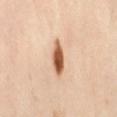This lesion was catalogued during total-body skin photography and was not selected for biopsy.
The lesion is located on the right thigh.
The tile uses cross-polarized illumination.
A female patient, aged 38 to 42.
Automated image analysis of the tile measured an outline eccentricity of about 0.9 (0 = round, 1 = elongated) and a symmetry-axis asymmetry near 0.25. It also reported peripheral color asymmetry of about 2. And it measured a classifier nevus-likeness of about 100/100 and lesion-presence confidence of about 100/100.
The recorded lesion diameter is about 4 mm.
A close-up tile cropped from a whole-body skin photograph, about 15 mm across.